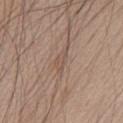Impression: Part of a total-body skin-imaging series; this lesion was reviewed on a skin check and was not flagged for biopsy. Clinical summary: A male subject, aged 63 to 67. This is a white-light tile. The total-body-photography lesion software estimated about 6 CIELAB-L* units darker than the surrounding skin. The analysis additionally found a border-irregularity index near 4/10, a color-variation rating of about 1/10, and a peripheral color-asymmetry measure near 0.5. It also reported an automated nevus-likeness rating near 0 out of 100. A lesion tile, about 15 mm wide, cut from a 3D total-body photograph. The lesion is on the chest.